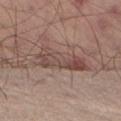  biopsy_status: not biopsied; imaged during a skin examination
  image:
    source: total-body photography crop
    field_of_view_mm: 15
  lighting: cross-polarized
  site: leg
  lesion_size:
    long_diameter_mm_approx: 7.0
  patient:
    sex: male
    age_approx: 60